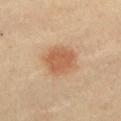Impression:
The lesion was tiled from a total-body skin photograph and was not biopsied.
Clinical summary:
Located on the front of the torso. Measured at roughly 4 mm in maximum diameter. The subject is a female aged around 40. A close-up tile cropped from a whole-body skin photograph, about 15 mm across.